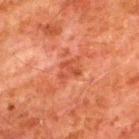The lesion was photographed on a routine skin check and not biopsied; there is no pathology result.
The total-body-photography lesion software estimated a mean CIELAB color near L≈42 a*≈29 b*≈32, about 6 CIELAB-L* units darker than the surrounding skin, and a normalized border contrast of about 5.5. It also reported a border-irregularity index near 3/10 and radial color variation of about 1.
The lesion is located on the upper back.
The recorded lesion diameter is about 3 mm.
The subject is a male aged approximately 80.
Captured under cross-polarized illumination.
A 15 mm close-up tile from a total-body photography series done for melanoma screening.